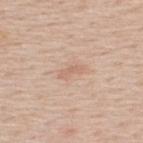Q: Is there a histopathology result?
A: total-body-photography surveillance lesion; no biopsy
Q: What is the lesion's diameter?
A: about 3 mm
Q: What is the anatomic site?
A: the upper back
Q: What lighting was used for the tile?
A: white-light illumination
Q: How was this image acquired?
A: ~15 mm crop, total-body skin-cancer survey
Q: Patient demographics?
A: male, aged 58 to 62